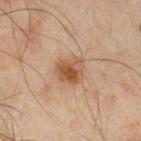Located on the leg. A roughly 15 mm field-of-view crop from a total-body skin photograph. The patient is a male approximately 45 years of age.